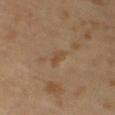| feature | finding |
|---|---|
| notes | no biopsy performed (imaged during a skin exam) |
| tile lighting | cross-polarized |
| lesion diameter | ~2.5 mm (longest diameter) |
| subject | male, approximately 65 years of age |
| image-analysis metrics | roughly 6 lightness units darker than nearby skin and a normalized border contrast of about 5.5; border irregularity of about 3 on a 0–10 scale, a within-lesion color-variation index near 0.5/10, and peripheral color asymmetry of about 0; lesion-presence confidence of about 100/100 |
| imaging modality | ~15 mm tile from a whole-body skin photo |